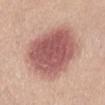Imaged during a routine full-body skin examination; the lesion was not biopsied and no histopathology is available. The lesion is on the abdomen. A roughly 15 mm field-of-view crop from a total-body skin photograph. A female patient about 50 years old. The lesion-visualizer software estimated an area of roughly 43 mm², a shape eccentricity near 0.6, and a shape-asymmetry score of about 0.15 (0 = symmetric). It also reported a lesion color around L≈57 a*≈24 b*≈24 in CIELAB, a lesion–skin lightness drop of about 15, and a normalized border contrast of about 9.5. And it measured an automated nevus-likeness rating near 100 out of 100 and lesion-presence confidence of about 100/100. Imaged with white-light lighting. Measured at roughly 9 mm in maximum diameter.